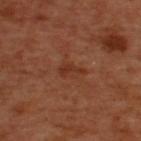<record>
<biopsy_status>not biopsied; imaged during a skin examination</biopsy_status>
<patient>
  <sex>male</sex>
  <age_approx>50</age_approx>
</patient>
<site>upper back</site>
<image>
  <source>total-body photography crop</source>
  <field_of_view_mm>15</field_of_view_mm>
</image>
</record>